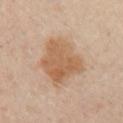The lesion was tiled from a total-body skin photograph and was not biopsied.
The subject is about 55 years old.
Located on the front of the torso.
A lesion tile, about 15 mm wide, cut from a 3D total-body photograph.
Longest diameter approximately 6 mm.
The tile uses cross-polarized illumination.